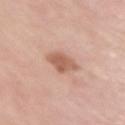The tile uses white-light illumination. A female subject in their 60s. The lesion's longest dimension is about 3 mm. Cropped from a total-body skin-imaging series; the visible field is about 15 mm. The lesion is on the right upper arm.